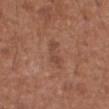Clinical impression: The lesion was photographed on a routine skin check and not biopsied; there is no pathology result. Context: From the abdomen. A male patient, in their mid-60s. A region of skin cropped from a whole-body photographic capture, roughly 15 mm wide.